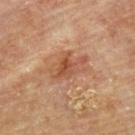Impression:
No biopsy was performed on this lesion — it was imaged during a full skin examination and was not determined to be concerning.
Image and clinical context:
From the upper back. Captured under cross-polarized illumination. The subject is a male about 85 years old or older. Cropped from a total-body skin-imaging series; the visible field is about 15 mm.